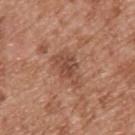workup: no biopsy performed (imaged during a skin exam)
site: the upper back
image source: total-body-photography crop, ~15 mm field of view
TBP lesion metrics: an area of roughly 8 mm² and an outline eccentricity of about 0.75 (0 = round, 1 = elongated); a border-irregularity rating of about 5/10 and a peripheral color-asymmetry measure near 1; a detector confidence of about 100 out of 100 that the crop contains a lesion
patient: male, in their mid-50s
lesion size: about 4 mm